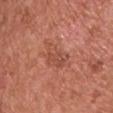Imaged during a routine full-body skin examination; the lesion was not biopsied and no histopathology is available. On the chest. Approximately 4 mm at its widest. A region of skin cropped from a whole-body photographic capture, roughly 15 mm wide. The subject is a male in their mid-50s. The lesion-visualizer software estimated a footprint of about 7 mm², an eccentricity of roughly 0.85, and a symmetry-axis asymmetry near 0.3. The analysis additionally found a classifier nevus-likeness of about 5/100 and a lesion-detection confidence of about 100/100. Imaged with white-light lighting.